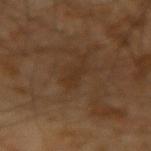Notes:
• follow-up: no biopsy performed (imaged during a skin exam)
• patient: male, in their mid- to late 60s
• image: ~15 mm tile from a whole-body skin photo
• TBP lesion metrics: a lesion color around L≈30 a*≈16 b*≈28 in CIELAB, roughly 4 lightness units darker than nearby skin, and a normalized border contrast of about 5
• lesion size: ~2.5 mm (longest diameter)
• lighting: cross-polarized